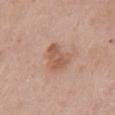<tbp_lesion>
  <biopsy_status>not biopsied; imaged during a skin examination</biopsy_status>
  <image>
    <source>total-body photography crop</source>
    <field_of_view_mm>15</field_of_view_mm>
  </image>
  <lesion_size>
    <long_diameter_mm_approx>4.0</long_diameter_mm_approx>
  </lesion_size>
  <site>chest</site>
  <lighting>white-light</lighting>
  <patient>
    <sex>female</sex>
    <age_approx>65</age_approx>
  </patient>
</tbp_lesion>